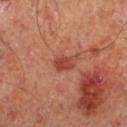Part of a total-body skin-imaging series; this lesion was reviewed on a skin check and was not flagged for biopsy. This is a cross-polarized tile. An algorithmic analysis of the crop reported a shape eccentricity near 0.75 and two-axis asymmetry of about 0.25. The software also gave roughly 10 lightness units darker than nearby skin and a lesion-to-skin contrast of about 7.5 (normalized; higher = more distinct). The analysis additionally found a nevus-likeness score of about 25/100. From the right lower leg. Measured at roughly 2.5 mm in maximum diameter. A male subject, roughly 65 years of age. Cropped from a total-body skin-imaging series; the visible field is about 15 mm.